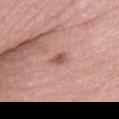biopsy status: imaged on a skin check; not biopsied.